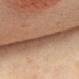Q: Is there a histopathology result?
A: total-body-photography surveillance lesion; no biopsy
Q: What kind of image is this?
A: ~15 mm crop, total-body skin-cancer survey
Q: Where on the body is the lesion?
A: the front of the torso
Q: What is the lesion's diameter?
A: ~1.5 mm (longest diameter)
Q: What are the patient's age and sex?
A: female, approximately 65 years of age